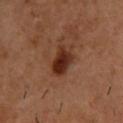Imaged during a routine full-body skin examination; the lesion was not biopsied and no histopathology is available. A male subject aged approximately 55. Automated tile analysis of the lesion measured an area of roughly 8 mm² and a shape eccentricity near 0.7. And it measured a border-irregularity rating of about 2.5/10 and a within-lesion color-variation index near 4.5/10. The analysis additionally found an automated nevus-likeness rating near 95 out of 100 and a detector confidence of about 100 out of 100 that the crop contains a lesion. Located on the upper back. Longest diameter approximately 4 mm. Cropped from a total-body skin-imaging series; the visible field is about 15 mm.